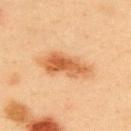Part of a total-body skin-imaging series; this lesion was reviewed on a skin check and was not flagged for biopsy.
A male patient, in their 40s.
A roughly 15 mm field-of-view crop from a total-body skin photograph.
The recorded lesion diameter is about 6.5 mm.
The tile uses cross-polarized illumination.
From the back.